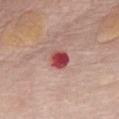Impression: This lesion was catalogued during total-body skin photography and was not selected for biopsy. Acquisition and patient details: A female patient, approximately 65 years of age. About 2.5 mm across. This image is a 15 mm lesion crop taken from a total-body photograph. The lesion is located on the chest.